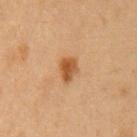Background: From the left upper arm. A female subject aged around 40. The total-body-photography lesion software estimated a footprint of about 5 mm², a shape eccentricity near 0.7, and two-axis asymmetry of about 0.25. It also reported a lesion color around L≈46 a*≈21 b*≈36 in CIELAB and roughly 11 lightness units darker than nearby skin. It also reported border irregularity of about 2 on a 0–10 scale, a color-variation rating of about 3/10, and a peripheral color-asymmetry measure near 1. Captured under cross-polarized illumination. A region of skin cropped from a whole-body photographic capture, roughly 15 mm wide. The recorded lesion diameter is about 3 mm.